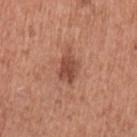Case summary:
– follow-up: catalogued during a skin exam; not biopsied
– patient: female, roughly 50 years of age
– image: 15 mm crop, total-body photography
– tile lighting: white-light illumination
– anatomic site: the left upper arm